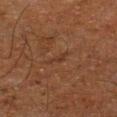The lesion was photographed on a routine skin check and not biopsied; there is no pathology result. A male subject aged approximately 65. Automated tile analysis of the lesion measured a footprint of about 2.5 mm². The software also gave a lesion color around L≈28 a*≈18 b*≈25 in CIELAB and a normalized border contrast of about 4.5. It also reported border irregularity of about 4.5 on a 0–10 scale, internal color variation of about 0 on a 0–10 scale, and peripheral color asymmetry of about 0. It also reported a nevus-likeness score of about 0/100. The tile uses cross-polarized illumination. A region of skin cropped from a whole-body photographic capture, roughly 15 mm wide. The lesion is on the leg. Measured at roughly 2.5 mm in maximum diameter.